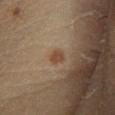follow-up = total-body-photography surveillance lesion; no biopsy | patient = male, about 60 years old | location = the leg | imaging modality = ~15 mm tile from a whole-body skin photo.